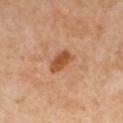Notes:
– notes · no biopsy performed (imaged during a skin exam)
– lesion diameter · about 3.5 mm
– anatomic site · the left lower leg
– image-analysis metrics · a lesion area of about 5 mm², an outline eccentricity of about 0.85 (0 = round, 1 = elongated), and a shape-asymmetry score of about 0.25 (0 = symmetric); border irregularity of about 2.5 on a 0–10 scale and a color-variation rating of about 2.5/10
– lighting · cross-polarized
– imaging modality · ~15 mm tile from a whole-body skin photo
– patient · female, approximately 50 years of age